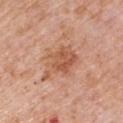Part of a total-body skin-imaging series; this lesion was reviewed on a skin check and was not flagged for biopsy.